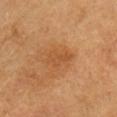The lesion was tiled from a total-body skin photograph and was not biopsied. Located on the head or neck. This image is a 15 mm lesion crop taken from a total-body photograph. The subject is a female roughly 55 years of age. Measured at roughly 3.5 mm in maximum diameter.